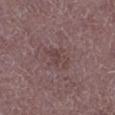Findings:
• acquisition · ~15 mm tile from a whole-body skin photo
• lighting · white-light illumination
• anatomic site · the left lower leg
• subject · male, aged approximately 50
• diameter · ≈3.5 mm